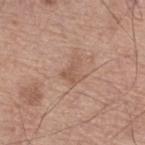Assessment:
Part of a total-body skin-imaging series; this lesion was reviewed on a skin check and was not flagged for biopsy.
Context:
Captured under white-light illumination. The total-body-photography lesion software estimated a lesion area of about 4 mm² and a shape eccentricity near 0.65. The software also gave an automated nevus-likeness rating near 0 out of 100 and a lesion-detection confidence of about 100/100. Approximately 3 mm at its widest. A roughly 15 mm field-of-view crop from a total-body skin photograph. A male patient in their mid- to late 60s. The lesion is located on the leg.